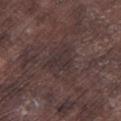Q: Was this lesion biopsied?
A: imaged on a skin check; not biopsied
Q: Lesion location?
A: the left lower leg
Q: How was the tile lit?
A: white-light illumination
Q: What are the patient's age and sex?
A: male, aged approximately 75
Q: How was this image acquired?
A: total-body-photography crop, ~15 mm field of view